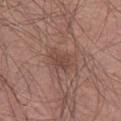Assessment:
This lesion was catalogued during total-body skin photography and was not selected for biopsy.
Acquisition and patient details:
A male subject, aged 53 to 57. Located on the left thigh. A lesion tile, about 15 mm wide, cut from a 3D total-body photograph.